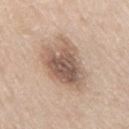Q: Is there a histopathology result?
A: no biopsy performed (imaged during a skin exam)
Q: Who is the patient?
A: male, aged approximately 65
Q: Where on the body is the lesion?
A: the mid back
Q: Illumination type?
A: white-light
Q: Lesion size?
A: about 7.5 mm
Q: What kind of image is this?
A: ~15 mm crop, total-body skin-cancer survey